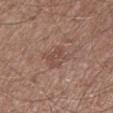No biopsy was performed on this lesion — it was imaged during a full skin examination and was not determined to be concerning. A male subject, about 65 years old. A lesion tile, about 15 mm wide, cut from a 3D total-body photograph. The lesion is located on the left lower leg.